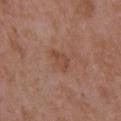An algorithmic analysis of the crop reported a lesion area of about 4.5 mm², a shape eccentricity near 0.85, and a shape-asymmetry score of about 0.35 (0 = symmetric). It also reported a lesion color around L≈46 a*≈21 b*≈30 in CIELAB and roughly 7 lightness units darker than nearby skin. The analysis additionally found a within-lesion color-variation index near 1.5/10.
A female patient aged 33–37.
A region of skin cropped from a whole-body photographic capture, roughly 15 mm wide.
Imaged with white-light lighting.
From the left upper arm.
Longest diameter approximately 3 mm.